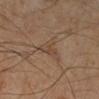notes: imaged on a skin check; not biopsied | TBP lesion metrics: a classifier nevus-likeness of about 0/100 and a lesion-detection confidence of about 85/100 | subject: male, aged approximately 60 | diameter: about 3.5 mm | acquisition: ~15 mm tile from a whole-body skin photo | site: the left lower leg | lighting: cross-polarized illumination.